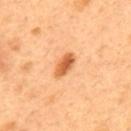Findings:
- tile lighting — cross-polarized illumination
- subject — male, approximately 50 years of age
- diameter — ≈3.5 mm
- image-analysis metrics — a footprint of about 5 mm², an eccentricity of roughly 0.85, and a shape-asymmetry score of about 0.15 (0 = symmetric); a lesion–skin lightness drop of about 15; border irregularity of about 1.5 on a 0–10 scale, internal color variation of about 4.5 on a 0–10 scale, and radial color variation of about 1.5; a classifier nevus-likeness of about 95/100 and a lesion-detection confidence of about 100/100
- site — the upper back
- image source — ~15 mm tile from a whole-body skin photo